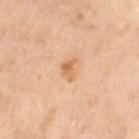site: left thigh
image:
  source: total-body photography crop
  field_of_view_mm: 15
lighting: cross-polarized
patient:
  sex: female
  age_approx: 55